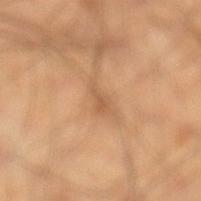Clinical impression: This lesion was catalogued during total-body skin photography and was not selected for biopsy. Background: Located on the left lower leg. A 15 mm close-up tile from a total-body photography series done for melanoma screening. A male subject, aged approximately 40.